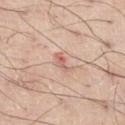Q: What is the imaging modality?
A: total-body-photography crop, ~15 mm field of view
Q: What is the anatomic site?
A: the left thigh
Q: How large is the lesion?
A: ~3 mm (longest diameter)
Q: How was the tile lit?
A: white-light illumination
Q: What are the patient's age and sex?
A: male, in their 70s